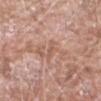Clinical impression: Imaged during a routine full-body skin examination; the lesion was not biopsied and no histopathology is available. Context: The subject is a male approximately 60 years of age. From the left forearm. This image is a 15 mm lesion crop taken from a total-body photograph. This is a white-light tile.